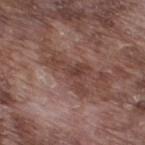Captured during whole-body skin photography for melanoma surveillance; the lesion was not biopsied. The patient is a male in their mid-70s. On the right thigh. A roughly 15 mm field-of-view crop from a total-body skin photograph. The lesion-visualizer software estimated border irregularity of about 8.5 on a 0–10 scale, internal color variation of about 3.5 on a 0–10 scale, and peripheral color asymmetry of about 1. The analysis additionally found a nevus-likeness score of about 0/100 and a lesion-detection confidence of about 60/100. Longest diameter approximately 5.5 mm. This is a white-light tile.